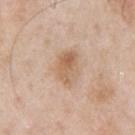follow-up = catalogued during a skin exam; not biopsied
lighting = white-light
TBP lesion metrics = a shape eccentricity near 0.75 and two-axis asymmetry of about 0.2; internal color variation of about 6 on a 0–10 scale and peripheral color asymmetry of about 2
anatomic site = the left upper arm
imaging modality = total-body-photography crop, ~15 mm field of view
subject = male, aged 53–57